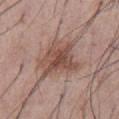| feature | finding |
|---|---|
| workup | total-body-photography surveillance lesion; no biopsy |
| location | the abdomen |
| automated lesion analysis | a lesion area of about 18 mm², an outline eccentricity of about 0.5 (0 = round, 1 = elongated), and a symmetry-axis asymmetry near 0.4; a lesion color around L≈50 a*≈19 b*≈24 in CIELAB and about 10 CIELAB-L* units darker than the surrounding skin; internal color variation of about 5 on a 0–10 scale; a nevus-likeness score of about 70/100 and a detector confidence of about 100 out of 100 that the crop contains a lesion |
| lighting | white-light |
| image | total-body-photography crop, ~15 mm field of view |
| patient | male, aged around 55 |
| diameter | ≈6.5 mm |